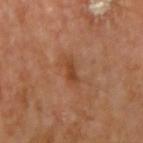{
  "biopsy_status": "not biopsied; imaged during a skin examination",
  "image": {
    "source": "total-body photography crop",
    "field_of_view_mm": 15
  },
  "lesion_size": {
    "long_diameter_mm_approx": 3.0
  },
  "site": "arm"
}